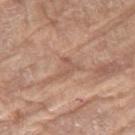Assessment:
This lesion was catalogued during total-body skin photography and was not selected for biopsy.
Background:
A female subject, aged approximately 75. The lesion-visualizer software estimated an area of roughly 3 mm², a shape eccentricity near 0.7, and a shape-asymmetry score of about 0.7 (0 = symmetric). And it measured a mean CIELAB color near L≈55 a*≈20 b*≈28, a lesion–skin lightness drop of about 7, and a lesion-to-skin contrast of about 5 (normalized; higher = more distinct). And it measured a within-lesion color-variation index near 0/10 and peripheral color asymmetry of about 0. The lesion is located on the right thigh. A 15 mm close-up tile from a total-body photography series done for melanoma screening.